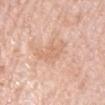Notes:
• notes · catalogued during a skin exam; not biopsied
• imaging modality · 15 mm crop, total-body photography
• patient · male, aged 78–82
• location · the mid back